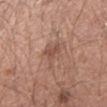Assessment: No biopsy was performed on this lesion — it was imaged during a full skin examination and was not determined to be concerning. Clinical summary: The tile uses white-light illumination. A male patient in their 30s. A roughly 15 mm field-of-view crop from a total-body skin photograph. Located on the arm. The lesion's longest dimension is about 4 mm.